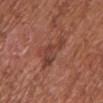Imaged during a routine full-body skin examination; the lesion was not biopsied and no histopathology is available. The lesion's longest dimension is about 5 mm. Cropped from a whole-body photographic skin survey; the tile spans about 15 mm. The lesion is located on the left upper arm. A female patient aged approximately 80. Imaged with white-light lighting.